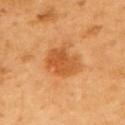<tbp_lesion>
<biopsy_status>not biopsied; imaged during a skin examination</biopsy_status>
<image>
  <source>total-body photography crop</source>
  <field_of_view_mm>15</field_of_view_mm>
</image>
<site>left upper arm</site>
<patient>
  <sex>male</sex>
  <age_approx>60</age_approx>
</patient>
</tbp_lesion>